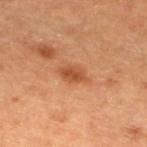  biopsy_status: not biopsied; imaged during a skin examination
  lesion_size:
    long_diameter_mm_approx: 3.5
  site: left lower leg
  image:
    source: total-body photography crop
    field_of_view_mm: 15
  patient:
    sex: female
    age_approx: 60
  lighting: cross-polarized